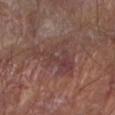Assessment: The lesion was tiled from a total-body skin photograph and was not biopsied. Context: The recorded lesion diameter is about 6.5 mm. Located on the right forearm. A roughly 15 mm field-of-view crop from a total-body skin photograph. A male patient in their mid- to late 80s or older. Captured under white-light illumination.